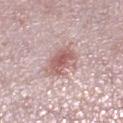Assessment: The lesion was photographed on a routine skin check and not biopsied; there is no pathology result. Acquisition and patient details: A female patient approximately 40 years of age. A 15 mm close-up extracted from a 3D total-body photography capture. Approximately 4 mm at its widest. Captured under white-light illumination. The total-body-photography lesion software estimated about 12 CIELAB-L* units darker than the surrounding skin and a normalized border contrast of about 7.5. And it measured a border-irregularity rating of about 2.5/10, a color-variation rating of about 4.5/10, and a peripheral color-asymmetry measure near 1.5. Located on the right lower leg.